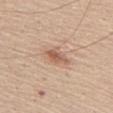The patient is a male approximately 65 years of age.
Imaged with white-light lighting.
Measured at roughly 3 mm in maximum diameter.
A 15 mm crop from a total-body photograph taken for skin-cancer surveillance.
The lesion-visualizer software estimated an average lesion color of about L≈58 a*≈21 b*≈30 (CIELAB), roughly 11 lightness units darker than nearby skin, and a lesion-to-skin contrast of about 7 (normalized; higher = more distinct).
The lesion is located on the upper back.